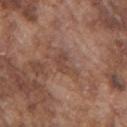Captured during whole-body skin photography for melanoma surveillance; the lesion was not biopsied. This image is a 15 mm lesion crop taken from a total-body photograph. The lesion is on the right upper arm. A male subject, aged around 75.